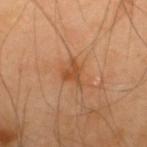A male subject, roughly 40 years of age. The lesion is located on the upper back. A 15 mm crop from a total-body photograph taken for skin-cancer surveillance. Automated tile analysis of the lesion measured a footprint of about 4.5 mm², an eccentricity of roughly 0.6, and two-axis asymmetry of about 0.5. It also reported a mean CIELAB color near L≈50 a*≈24 b*≈38 and a normalized border contrast of about 7.